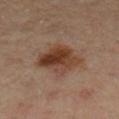- workup · no biopsy performed (imaged during a skin exam)
- site · the left leg
- image source · 15 mm crop, total-body photography
- patient · female, aged 58 to 62